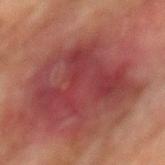Imaged during a routine full-body skin examination; the lesion was not biopsied and no histopathology is available. From the mid back. The subject is a male aged approximately 75. Cropped from a whole-body photographic skin survey; the tile spans about 15 mm.